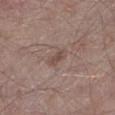Clinical impression:
This lesion was catalogued during total-body skin photography and was not selected for biopsy.
Image and clinical context:
The lesion is located on the left lower leg. A 15 mm close-up extracted from a 3D total-body photography capture. Measured at roughly 3 mm in maximum diameter. The lesion-visualizer software estimated a lesion area of about 4 mm² and an outline eccentricity of about 0.85 (0 = round, 1 = elongated). The analysis additionally found a border-irregularity rating of about 3/10, a within-lesion color-variation index near 1.5/10, and a peripheral color-asymmetry measure near 0.5. A male subject, aged 63–67. This is a white-light tile.